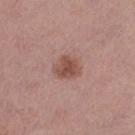The lesion was tiled from a total-body skin photograph and was not biopsied. A lesion tile, about 15 mm wide, cut from a 3D total-body photograph. The lesion is located on the left lower leg. The subject is a female aged around 40. The recorded lesion diameter is about 3 mm. This is a white-light tile.